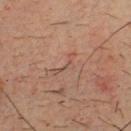* workup · catalogued during a skin exam; not biopsied
* subject · male, aged approximately 35
* lighting · cross-polarized
* image source · total-body-photography crop, ~15 mm field of view
* anatomic site · the chest
* size · ~4 mm (longest diameter)